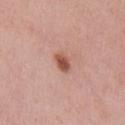Q: Was a biopsy performed?
A: imaged on a skin check; not biopsied
Q: What lighting was used for the tile?
A: white-light illumination
Q: How was this image acquired?
A: total-body-photography crop, ~15 mm field of view
Q: What did automated image analysis measure?
A: a lesion area of about 4 mm², an eccentricity of roughly 0.75, and a symmetry-axis asymmetry near 0.2; border irregularity of about 1.5 on a 0–10 scale, a within-lesion color-variation index near 3/10, and peripheral color asymmetry of about 1; an automated nevus-likeness rating near 95 out of 100 and a detector confidence of about 100 out of 100 that the crop contains a lesion
Q: Who is the patient?
A: male, approximately 60 years of age
Q: What is the anatomic site?
A: the chest
Q: How large is the lesion?
A: about 2.5 mm